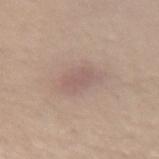workup: catalogued during a skin exam; not biopsied | body site: the arm | lighting: white-light illumination | lesion diameter: ~4 mm (longest diameter) | patient: female, roughly 45 years of age | image source: 15 mm crop, total-body photography.